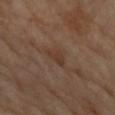notes — total-body-photography surveillance lesion; no biopsy | anatomic site — the left forearm | automated metrics — a lesion color around L≈36 a*≈17 b*≈27 in CIELAB and a normalized lesion–skin contrast near 6 | patient — female, aged 68 to 72 | lesion size — ≈2.5 mm | image source — 15 mm crop, total-body photography | lighting — cross-polarized.